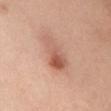Q: Is there a histopathology result?
A: imaged on a skin check; not biopsied